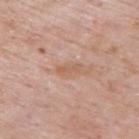| feature | finding |
|---|---|
| lesion diameter | about 3 mm |
| location | the upper back |
| lighting | white-light |
| patient | male, roughly 55 years of age |
| image source | ~15 mm tile from a whole-body skin photo |